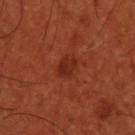The lesion was tiled from a total-body skin photograph and was not biopsied.
The recorded lesion diameter is about 2.5 mm.
A roughly 15 mm field-of-view crop from a total-body skin photograph.
The lesion is on the head or neck.
A male subject, aged 53–57.
The tile uses cross-polarized illumination.